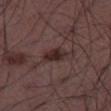Q: Was this lesion biopsied?
A: imaged on a skin check; not biopsied
Q: How was the tile lit?
A: white-light illumination
Q: How was this image acquired?
A: 15 mm crop, total-body photography
Q: Patient demographics?
A: male, approximately 50 years of age
Q: What is the anatomic site?
A: the left thigh